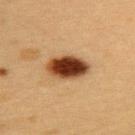Case summary:
* biopsy status · catalogued during a skin exam; not biopsied
* lesion diameter · about 5 mm
* imaging modality · 15 mm crop, total-body photography
* subject · female, aged approximately 30
* anatomic site · the left upper arm